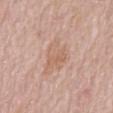- biopsy status — total-body-photography surveillance lesion; no biopsy
- body site — the mid back
- illumination — white-light illumination
- subject — female, roughly 80 years of age
- diameter — about 5 mm
- image source — 15 mm crop, total-body photography